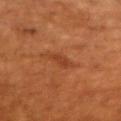workup: catalogued during a skin exam; not biopsied | subject: female, aged around 80 | location: the upper back | imaging modality: total-body-photography crop, ~15 mm field of view.